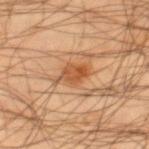{
  "biopsy_status": "not biopsied; imaged during a skin examination",
  "image": {
    "source": "total-body photography crop",
    "field_of_view_mm": 15
  },
  "lesion_size": {
    "long_diameter_mm_approx": 3.5
  },
  "site": "right lower leg",
  "lighting": "cross-polarized",
  "automated_metrics": {
    "area_mm2_approx": 6.5,
    "eccentricity": 0.8,
    "shape_asymmetry": 0.25,
    "cielab_L": 53,
    "cielab_a": 25,
    "cielab_b": 38,
    "vs_skin_darker_L": 11.0,
    "vs_skin_contrast_norm": 8.0
  },
  "patient": {
    "sex": "male",
    "age_approx": 45
  }
}